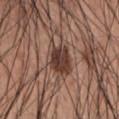workup=imaged on a skin check; not biopsied | automated metrics=an average lesion color of about L≈39 a*≈19 b*≈23 (CIELAB) and about 13 CIELAB-L* units darker than the surrounding skin; border irregularity of about 2.5 on a 0–10 scale, a within-lesion color-variation index near 4/10, and radial color variation of about 1.5; an automated nevus-likeness rating near 95 out of 100 and a lesion-detection confidence of about 100/100 | image source=15 mm crop, total-body photography | patient=male, about 55 years old | size=~4.5 mm (longest diameter) | body site=the abdomen.